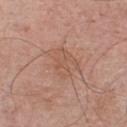| feature | finding |
|---|---|
| biopsy status | imaged on a skin check; not biopsied |
| subject | male, about 55 years old |
| acquisition | ~15 mm tile from a whole-body skin photo |
| location | the chest |
| tile lighting | white-light |